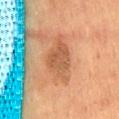Q: Was this lesion biopsied?
A: total-body-photography surveillance lesion; no biopsy
Q: What lighting was used for the tile?
A: cross-polarized illumination
Q: Where on the body is the lesion?
A: the back
Q: How large is the lesion?
A: ~6 mm (longest diameter)
Q: How was this image acquired?
A: total-body-photography crop, ~15 mm field of view
Q: Automated lesion metrics?
A: a footprint of about 14 mm², an outline eccentricity of about 0.85 (0 = round, 1 = elongated), and two-axis asymmetry of about 0.25; a nevus-likeness score of about 0/100 and a lesion-detection confidence of about 100/100
Q: Who is the patient?
A: male, in their mid-50s